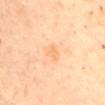Case summary:
- workup · catalogued during a skin exam; not biopsied
- automated lesion analysis · an average lesion color of about L≈75 a*≈22 b*≈42 (CIELAB), roughly 6 lightness units darker than nearby skin, and a normalized lesion–skin contrast near 4.5; an automated nevus-likeness rating near 0 out of 100 and lesion-presence confidence of about 100/100
- image source · 15 mm crop, total-body photography
- subject · female, aged approximately 65
- tile lighting · cross-polarized
- anatomic site · the mid back